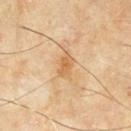{"biopsy_status": "not biopsied; imaged during a skin examination", "image": {"source": "total-body photography crop", "field_of_view_mm": 15}, "site": "mid back", "lesion_size": {"long_diameter_mm_approx": 3.0}, "patient": {"sex": "male", "age_approx": 60}, "lighting": "cross-polarized"}